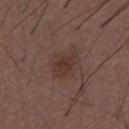Q: Was this lesion biopsied?
A: total-body-photography surveillance lesion; no biopsy
Q: Lesion size?
A: ~3.5 mm (longest diameter)
Q: What are the patient's age and sex?
A: male, aged 48–52
Q: Automated lesion metrics?
A: a shape eccentricity near 0.65; roughly 7 lightness units darker than nearby skin and a normalized lesion–skin contrast near 6.5; border irregularity of about 3 on a 0–10 scale and radial color variation of about 0.5; a nevus-likeness score of about 50/100 and a detector confidence of about 100 out of 100 that the crop contains a lesion
Q: What kind of image is this?
A: total-body-photography crop, ~15 mm field of view
Q: What is the anatomic site?
A: the front of the torso